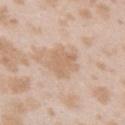Part of a total-body skin-imaging series; this lesion was reviewed on a skin check and was not flagged for biopsy.
A female subject about 25 years old.
A region of skin cropped from a whole-body photographic capture, roughly 15 mm wide.
The tile uses white-light illumination.
Approximately 4 mm at its widest.
The lesion is located on the right upper arm.